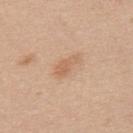Q: Is there a histopathology result?
A: imaged on a skin check; not biopsied